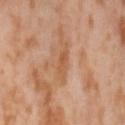Case summary:
– workup · total-body-photography surveillance lesion; no biopsy
– patient · female, aged approximately 55
– location · the lower back
– imaging modality · ~15 mm crop, total-body skin-cancer survey